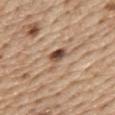Imaged with white-light lighting.
A male patient, aged approximately 70.
Cropped from a whole-body photographic skin survey; the tile spans about 15 mm.
The lesion is on the abdomen.
The total-body-photography lesion software estimated a mean CIELAB color near L≈50 a*≈18 b*≈29, about 14 CIELAB-L* units darker than the surrounding skin, and a normalized lesion–skin contrast near 10. It also reported a within-lesion color-variation index near 8/10 and radial color variation of about 2.5. And it measured a classifier nevus-likeness of about 65/100 and a lesion-detection confidence of about 100/100.